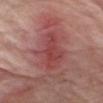Captured during whole-body skin photography for melanoma surveillance; the lesion was not biopsied. Longest diameter approximately 6.5 mm. The patient is a male aged 73 to 77. The lesion is on the right upper arm. A region of skin cropped from a whole-body photographic capture, roughly 15 mm wide.